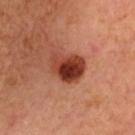Q: Was a biopsy performed?
A: total-body-photography surveillance lesion; no biopsy
Q: How was this image acquired?
A: total-body-photography crop, ~15 mm field of view
Q: Automated lesion metrics?
A: a within-lesion color-variation index near 9/10 and peripheral color asymmetry of about 3.5
Q: How was the tile lit?
A: cross-polarized illumination
Q: What is the lesion's diameter?
A: ~4 mm (longest diameter)
Q: Who is the patient?
A: female, aged 43 to 47
Q: Where on the body is the lesion?
A: the head or neck